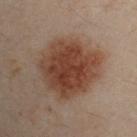Assessment: The lesion was tiled from a total-body skin photograph and was not biopsied. Acquisition and patient details: A male patient roughly 30 years of age. Automated image analysis of the tile measured a border-irregularity index near 2/10, a color-variation rating of about 4/10, and radial color variation of about 1. The analysis additionally found an automated nevus-likeness rating near 95 out of 100 and a detector confidence of about 100 out of 100 that the crop contains a lesion. On the arm. Imaged with cross-polarized lighting. Cropped from a total-body skin-imaging series; the visible field is about 15 mm. Longest diameter approximately 7 mm.